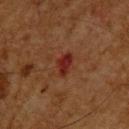Q: Is there a histopathology result?
A: imaged on a skin check; not biopsied
Q: What did automated image analysis measure?
A: a lesion area of about 4.5 mm², a shape eccentricity near 0.75, and a symmetry-axis asymmetry near 0.3; a mean CIELAB color near L≈23 a*≈25 b*≈24; a classifier nevus-likeness of about 0/100 and lesion-presence confidence of about 100/100
Q: How was this image acquired?
A: ~15 mm tile from a whole-body skin photo
Q: Where on the body is the lesion?
A: the head or neck
Q: Illumination type?
A: cross-polarized
Q: Lesion size?
A: about 3 mm
Q: Who is the patient?
A: male, aged approximately 65